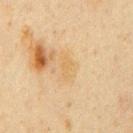biopsy status = total-body-photography surveillance lesion; no biopsy | diameter = ~2.5 mm (longest diameter) | location = the chest | tile lighting = cross-polarized illumination | image = ~15 mm crop, total-body skin-cancer survey | patient = male, about 60 years old.